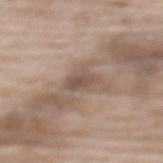Q: Was this lesion biopsied?
A: catalogued during a skin exam; not biopsied
Q: What are the patient's age and sex?
A: female, aged approximately 75
Q: Lesion size?
A: about 3 mm
Q: What lighting was used for the tile?
A: white-light
Q: What did automated image analysis measure?
A: a footprint of about 5 mm² and an outline eccentricity of about 0.7 (0 = round, 1 = elongated); a lesion color around L≈51 a*≈15 b*≈24 in CIELAB, about 9 CIELAB-L* units darker than the surrounding skin, and a lesion-to-skin contrast of about 6.5 (normalized; higher = more distinct); an automated nevus-likeness rating near 0 out of 100
Q: What kind of image is this?
A: ~15 mm tile from a whole-body skin photo
Q: Lesion location?
A: the back